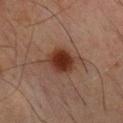This lesion was catalogued during total-body skin photography and was not selected for biopsy.